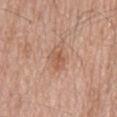Case summary:
• biopsy status — no biopsy performed (imaged during a skin exam)
• location — the mid back
• patient — male, about 80 years old
• lesion diameter — ~3.5 mm (longest diameter)
• image source — 15 mm crop, total-body photography
• lighting — white-light